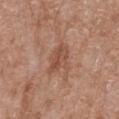follow-up — catalogued during a skin exam; not biopsied | subject — female, aged 73–77 | image — 15 mm crop, total-body photography | illumination — white-light illumination | diameter — about 3.5 mm | anatomic site — the right forearm | image-analysis metrics — a footprint of about 8 mm²; a lesion color around L≈51 a*≈22 b*≈30 in CIELAB, a lesion–skin lightness drop of about 8, and a normalized lesion–skin contrast near 6; a detector confidence of about 100 out of 100 that the crop contains a lesion.